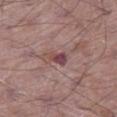Imaged during a routine full-body skin examination; the lesion was not biopsied and no histopathology is available.
A male subject, about 65 years old.
The lesion's longest dimension is about 2.5 mm.
This is a white-light tile.
The lesion is located on the right thigh.
A 15 mm close-up extracted from a 3D total-body photography capture.